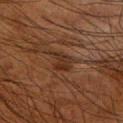Part of a total-body skin-imaging series; this lesion was reviewed on a skin check and was not flagged for biopsy. A male subject, in their mid-60s. Cropped from a whole-body photographic skin survey; the tile spans about 15 mm. The lesion's longest dimension is about 3.5 mm. Captured under cross-polarized illumination. Located on the right forearm.